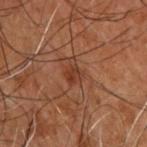Recorded during total-body skin imaging; not selected for excision or biopsy.